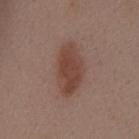{
  "biopsy_status": "not biopsied; imaged during a skin examination",
  "patient": {
    "sex": "female",
    "age_approx": 55
  },
  "image": {
    "source": "total-body photography crop",
    "field_of_view_mm": 15
  },
  "lesion_size": {
    "long_diameter_mm_approx": 6.0
  },
  "site": "front of the torso"
}